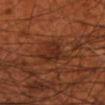Part of a total-body skin-imaging series; this lesion was reviewed on a skin check and was not flagged for biopsy. A close-up tile cropped from a whole-body skin photograph, about 15 mm across. The subject is a male about 70 years old. The lesion's longest dimension is about 3.5 mm. Imaged with cross-polarized lighting. The lesion is on the left forearm. Automated tile analysis of the lesion measured a lesion area of about 7.5 mm², a shape eccentricity near 0.7, and two-axis asymmetry of about 0.4. It also reported a mean CIELAB color near L≈27 a*≈23 b*≈28, a lesion–skin lightness drop of about 7, and a normalized border contrast of about 7.